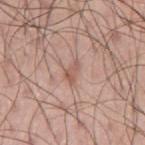Impression:
This lesion was catalogued during total-body skin photography and was not selected for biopsy.
Acquisition and patient details:
An algorithmic analysis of the crop reported an eccentricity of roughly 0.85 and a shape-asymmetry score of about 0.4 (0 = symmetric). The software also gave a lesion color around L≈56 a*≈20 b*≈27 in CIELAB and about 9 CIELAB-L* units darker than the surrounding skin. The analysis additionally found a border-irregularity rating of about 4/10, a within-lesion color-variation index near 0.5/10, and a peripheral color-asymmetry measure near 0. The lesion's longest dimension is about 2.5 mm. A close-up tile cropped from a whole-body skin photograph, about 15 mm across. A male patient, aged around 45. On the left upper arm.